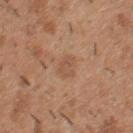Clinical impression: No biopsy was performed on this lesion — it was imaged during a full skin examination and was not determined to be concerning. Context: A male subject, approximately 40 years of age. Longest diameter approximately 2.5 mm. The lesion is on the back. Cropped from a total-body skin-imaging series; the visible field is about 15 mm. The total-body-photography lesion software estimated an eccentricity of roughly 0.45 and a symmetry-axis asymmetry near 0.2. And it measured a mean CIELAB color near L≈53 a*≈20 b*≈32, about 6 CIELAB-L* units darker than the surrounding skin, and a normalized lesion–skin contrast near 4.5. The software also gave a border-irregularity rating of about 2/10 and radial color variation of about 0.5. The software also gave a nevus-likeness score of about 0/100 and lesion-presence confidence of about 100/100. Imaged with white-light lighting.